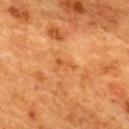| field | value |
|---|---|
| biopsy status | no biopsy performed (imaged during a skin exam) |
| subject | female, approximately 55 years of age |
| diameter | about 2.5 mm |
| body site | the upper back |
| tile lighting | cross-polarized illumination |
| automated lesion analysis | a footprint of about 2 mm², a shape eccentricity near 0.9, and a shape-asymmetry score of about 0.45 (0 = symmetric); a lesion color around L≈46 a*≈24 b*≈39 in CIELAB and a lesion–skin lightness drop of about 6 |
| acquisition | 15 mm crop, total-body photography |